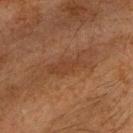  biopsy_status: not biopsied; imaged during a skin examination
  lesion_size:
    long_diameter_mm_approx: 4.5
  lighting: cross-polarized
  image:
    source: total-body photography crop
    field_of_view_mm: 15
  patient:
    sex: male
    age_approx: 65
  automated_metrics:
    area_mm2_approx: 6.5
    eccentricity: 0.95
    shape_asymmetry: 0.25
    vs_skin_contrast_norm: 5.5
    color_variation_0_10: 1.0
    peripheral_color_asymmetry: 0.5
    nevus_likeness_0_100: 5
    lesion_detection_confidence_0_100: 65
  site: head or neck